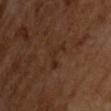{
  "biopsy_status": "not biopsied; imaged during a skin examination",
  "automated_metrics": {
    "area_mm2_approx": 4.0,
    "eccentricity": 0.8,
    "shape_asymmetry": 0.7,
    "cielab_L": 26,
    "cielab_a": 18,
    "cielab_b": 25,
    "vs_skin_darker_L": 5.0,
    "vs_skin_contrast_norm": 5.5
  },
  "lighting": "cross-polarized",
  "lesion_size": {
    "long_diameter_mm_approx": 3.5
  },
  "image": {
    "source": "total-body photography crop",
    "field_of_view_mm": 15
  },
  "patient": {
    "sex": "male",
    "age_approx": 65
  }
}